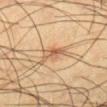follow-up: catalogued during a skin exam; not biopsied | subject: male, aged approximately 35 | lighting: cross-polarized illumination | image: ~15 mm crop, total-body skin-cancer survey | size: ≈3.5 mm | body site: the right lower leg.